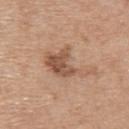Automated tile analysis of the lesion measured a border-irregularity rating of about 6.5/10, internal color variation of about 4 on a 0–10 scale, and a peripheral color-asymmetry measure near 1.5. The patient is a male aged 68 to 72. The tile uses white-light illumination. A close-up tile cropped from a whole-body skin photograph, about 15 mm across. The lesion is located on the upper back. The recorded lesion diameter is about 6 mm.